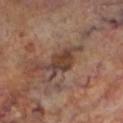notes: catalogued during a skin exam; not biopsied
body site: the left lower leg
subject: male, aged approximately 70
tile lighting: cross-polarized illumination
TBP lesion metrics: an average lesion color of about L≈38 a*≈18 b*≈24 (CIELAB), about 10 CIELAB-L* units darker than the surrounding skin, and a lesion-to-skin contrast of about 8.5 (normalized; higher = more distinct); a border-irregularity index near 3.5/10, a color-variation rating of about 4/10, and peripheral color asymmetry of about 1.5
lesion size: ≈4 mm
imaging modality: total-body-photography crop, ~15 mm field of view